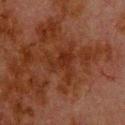{"biopsy_status": "not biopsied; imaged during a skin examination", "image": {"source": "total-body photography crop", "field_of_view_mm": 15}, "site": "upper back", "lighting": "cross-polarized", "patient": {"sex": "male", "age_approx": 80}, "lesion_size": {"long_diameter_mm_approx": 4.5}}